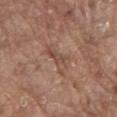This lesion was catalogued during total-body skin photography and was not selected for biopsy. Measured at roughly 4.5 mm in maximum diameter. Captured under white-light illumination. On the mid back. The subject is a male roughly 80 years of age. A 15 mm close-up tile from a total-body photography series done for melanoma screening.